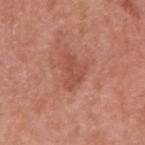Q: Is there a histopathology result?
A: no biopsy performed (imaged during a skin exam)
Q: Illumination type?
A: white-light illumination
Q: Lesion location?
A: the right upper arm
Q: Who is the patient?
A: male, roughly 70 years of age
Q: What did automated image analysis measure?
A: a footprint of about 5.5 mm², a shape eccentricity near 0.85, and a shape-asymmetry score of about 0.5 (0 = symmetric); border irregularity of about 5.5 on a 0–10 scale, a within-lesion color-variation index near 1.5/10, and peripheral color asymmetry of about 0.5; a classifier nevus-likeness of about 5/100 and a detector confidence of about 100 out of 100 that the crop contains a lesion
Q: What is the lesion's diameter?
A: about 4 mm
Q: How was this image acquired?
A: ~15 mm tile from a whole-body skin photo